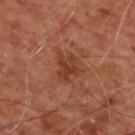Case summary:
- biopsy status — no biopsy performed (imaged during a skin exam)
- subject — male, aged 58–62
- diameter — ≈3.5 mm
- illumination — cross-polarized illumination
- location — the front of the torso
- image source — ~15 mm crop, total-body skin-cancer survey
- image-analysis metrics — a footprint of about 6.5 mm² and a shape-asymmetry score of about 0.4 (0 = symmetric); about 8 CIELAB-L* units darker than the surrounding skin and a normalized lesion–skin contrast near 6.5; a color-variation rating of about 2.5/10 and a peripheral color-asymmetry measure near 1